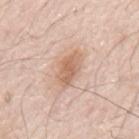| key | value |
|---|---|
| follow-up | no biopsy performed (imaged during a skin exam) |
| site | the mid back |
| subject | male, aged 78 to 82 |
| imaging modality | ~15 mm tile from a whole-body skin photo |